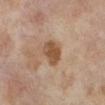| feature | finding |
|---|---|
| workup | no biopsy performed (imaged during a skin exam) |
| location | the leg |
| image | ~15 mm tile from a whole-body skin photo |
| lesion diameter | about 3.5 mm |
| patient | female, in their 50s |